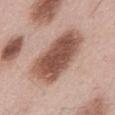Q: Was this lesion biopsied?
A: imaged on a skin check; not biopsied
Q: Patient demographics?
A: male, aged 53 to 57
Q: Lesion size?
A: about 7.5 mm
Q: Automated lesion metrics?
A: an average lesion color of about L≈53 a*≈21 b*≈27 (CIELAB), roughly 16 lightness units darker than nearby skin, and a lesion-to-skin contrast of about 11 (normalized; higher = more distinct); an automated nevus-likeness rating near 35 out of 100 and a detector confidence of about 100 out of 100 that the crop contains a lesion
Q: Illumination type?
A: white-light
Q: Where on the body is the lesion?
A: the mid back
Q: What is the imaging modality?
A: ~15 mm tile from a whole-body skin photo